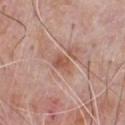Q: Automated lesion metrics?
A: a footprint of about 4 mm², an eccentricity of roughly 0.65, and two-axis asymmetry of about 0.2; a border-irregularity index near 1.5/10 and radial color variation of about 1
Q: What is the lesion's diameter?
A: about 2.5 mm
Q: Who is the patient?
A: male, approximately 70 years of age
Q: What kind of image is this?
A: ~15 mm crop, total-body skin-cancer survey
Q: Where on the body is the lesion?
A: the front of the torso